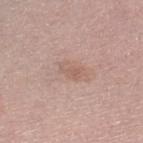* notes · catalogued during a skin exam; not biopsied
* size · ~3 mm (longest diameter)
* acquisition · total-body-photography crop, ~15 mm field of view
* site · the right lower leg
* automated lesion analysis · an average lesion color of about L≈59 a*≈19 b*≈25 (CIELAB), about 7 CIELAB-L* units darker than the surrounding skin, and a lesion-to-skin contrast of about 5 (normalized; higher = more distinct); internal color variation of about 2.5 on a 0–10 scale and a peripheral color-asymmetry measure near 0.5
* patient · female, approximately 65 years of age